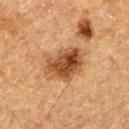Impression: The lesion was photographed on a routine skin check and not biopsied; there is no pathology result. Clinical summary: A male patient aged 73 to 77. The recorded lesion diameter is about 5.5 mm. The lesion is on the left thigh. Imaged with cross-polarized lighting. A lesion tile, about 15 mm wide, cut from a 3D total-body photograph.